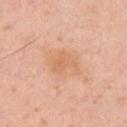Imaged during a routine full-body skin examination; the lesion was not biopsied and no histopathology is available.
A region of skin cropped from a whole-body photographic capture, roughly 15 mm wide.
The lesion is located on the right upper arm.
The tile uses cross-polarized illumination.
The lesion-visualizer software estimated about 6 CIELAB-L* units darker than the surrounding skin and a lesion-to-skin contrast of about 5 (normalized; higher = more distinct). And it measured a border-irregularity rating of about 4/10 and a peripheral color-asymmetry measure near 1. And it measured a classifier nevus-likeness of about 10/100 and a lesion-detection confidence of about 100/100.
Approximately 4 mm at its widest.
A male subject, aged approximately 45.